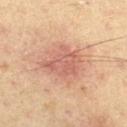Q: What kind of image is this?
A: 15 mm crop, total-body photography
Q: How was the tile lit?
A: cross-polarized illumination
Q: What is the anatomic site?
A: the left thigh
Q: Patient demographics?
A: male, aged approximately 65